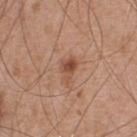Captured during whole-body skin photography for melanoma surveillance; the lesion was not biopsied. A male subject, about 55 years old. Located on the chest. This image is a 15 mm lesion crop taken from a total-body photograph. Longest diameter approximately 3 mm. Imaged with white-light lighting.